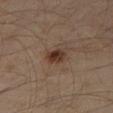Recorded during total-body skin imaging; not selected for excision or biopsy. A 15 mm close-up tile from a total-body photography series done for melanoma screening. Located on the right thigh. A male patient, roughly 60 years of age. The total-body-photography lesion software estimated an area of roughly 5 mm², a shape eccentricity near 0.6, and a symmetry-axis asymmetry near 0.2. And it measured about 10 CIELAB-L* units darker than the surrounding skin and a lesion-to-skin contrast of about 9.5 (normalized; higher = more distinct). It also reported a border-irregularity index near 2/10, a within-lesion color-variation index near 4/10, and a peripheral color-asymmetry measure near 1.5. The analysis additionally found a nevus-likeness score of about 95/100 and a lesion-detection confidence of about 100/100.